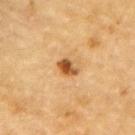Impression:
Captured during whole-body skin photography for melanoma surveillance; the lesion was not biopsied.
Context:
A male subject, in their mid- to late 80s or older. A roughly 15 mm field-of-view crop from a total-body skin photograph. The total-body-photography lesion software estimated an average lesion color of about L≈47 a*≈21 b*≈38 (CIELAB), a lesion–skin lightness drop of about 14, and a lesion-to-skin contrast of about 10 (normalized; higher = more distinct). And it measured lesion-presence confidence of about 100/100. The lesion is on the left upper arm.